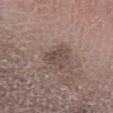Case summary:
- follow-up — imaged on a skin check; not biopsied
- tile lighting — white-light
- TBP lesion metrics — a lesion color around L≈46 a*≈15 b*≈19 in CIELAB, a lesion–skin lightness drop of about 9, and a normalized lesion–skin contrast near 6.5; lesion-presence confidence of about 100/100
- diameter — about 3.5 mm
- imaging modality — ~15 mm crop, total-body skin-cancer survey
- subject — male, in their 70s
- location — the left lower leg